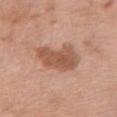Findings:
- workup · imaged on a skin check; not biopsied
- size · ≈5.5 mm
- automated lesion analysis · an average lesion color of about L≈55 a*≈22 b*≈31 (CIELAB), roughly 11 lightness units darker than nearby skin, and a normalized border contrast of about 8
- acquisition · 15 mm crop, total-body photography
- patient · female, aged 73 to 77
- location · the arm